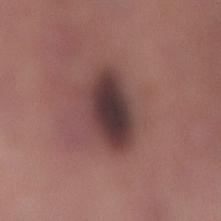{"biopsy_status": "not biopsied; imaged during a skin examination", "site": "right lower leg", "image": {"source": "total-body photography crop", "field_of_view_mm": 15}, "patient": {"sex": "female", "age_approx": 40}, "lesion_size": {"long_diameter_mm_approx": 6.0}, "automated_metrics": {"eccentricity": 0.85, "shape_asymmetry": 0.2, "color_variation_0_10": 6.5, "peripheral_color_asymmetry": 1.5, "nevus_likeness_0_100": 5, "lesion_detection_confidence_0_100": 95}}